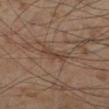Clinical impression:
The lesion was photographed on a routine skin check and not biopsied; there is no pathology result.
Image and clinical context:
On the left lower leg. A close-up tile cropped from a whole-body skin photograph, about 15 mm across. A male subject aged 53 to 57. About 3.5 mm across.